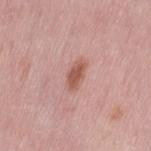Assessment: Imaged during a routine full-body skin examination; the lesion was not biopsied and no histopathology is available.